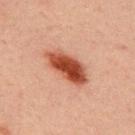Assessment:
This lesion was catalogued during total-body skin photography and was not selected for biopsy.
Image and clinical context:
Automated image analysis of the tile measured an outline eccentricity of about 0.9 (0 = round, 1 = elongated) and a symmetry-axis asymmetry near 0.25. The software also gave a lesion color around L≈41 a*≈26 b*≈30 in CIELAB, roughly 15 lightness units darker than nearby skin, and a normalized lesion–skin contrast near 12. And it measured a border-irregularity rating of about 2.5/10 and a within-lesion color-variation index near 5.5/10. Approximately 6 mm at its widest. On the upper back. Captured under cross-polarized illumination. A close-up tile cropped from a whole-body skin photograph, about 15 mm across. A male subject about 30 years old.